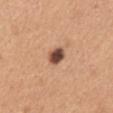Case summary:
* workup · total-body-photography surveillance lesion; no biopsy
* subject · female, aged around 30
* size · ≈2.5 mm
* body site · the mid back
* imaging modality · total-body-photography crop, ~15 mm field of view
* illumination · white-light